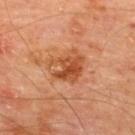Findings:
– notes: no biopsy performed (imaged during a skin exam)
– patient: male, aged around 65
– acquisition: 15 mm crop, total-body photography
– location: the upper back
– lesion diameter: about 4.5 mm